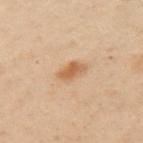Findings:
* biopsy status: catalogued during a skin exam; not biopsied
* size: ≈2.5 mm
* image source: ~15 mm tile from a whole-body skin photo
* tile lighting: cross-polarized
* anatomic site: the left upper arm
* subject: male, in their mid- to late 50s